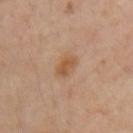An algorithmic analysis of the crop reported a footprint of about 5.5 mm², an eccentricity of roughly 0.7, and a shape-asymmetry score of about 0.2 (0 = symmetric). The analysis additionally found a mean CIELAB color near L≈54 a*≈19 b*≈34, roughly 8 lightness units darker than nearby skin, and a normalized lesion–skin contrast near 7. And it measured an automated nevus-likeness rating near 45 out of 100 and a lesion-detection confidence of about 100/100.
A female subject, approximately 45 years of age.
The recorded lesion diameter is about 3 mm.
This image is a 15 mm lesion crop taken from a total-body photograph.
This is a cross-polarized tile.
Located on the left upper arm.